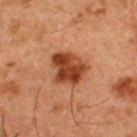notes: total-body-photography surveillance lesion; no biopsy
lesion size: ≈5 mm
automated lesion analysis: a footprint of about 14 mm² and an outline eccentricity of about 0.6 (0 = round, 1 = elongated); border irregularity of about 2.5 on a 0–10 scale, a within-lesion color-variation index near 5.5/10, and radial color variation of about 2; a nevus-likeness score of about 90/100 and a lesion-detection confidence of about 100/100
body site: the back
subject: male, aged around 50
illumination: cross-polarized
imaging modality: total-body-photography crop, ~15 mm field of view